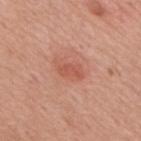follow-up: catalogued during a skin exam; not biopsied | size: ≈3 mm | automated lesion analysis: an area of roughly 3.5 mm², an outline eccentricity of about 0.85 (0 = round, 1 = elongated), and a shape-asymmetry score of about 0.35 (0 = symmetric); an average lesion color of about L≈55 a*≈29 b*≈31 (CIELAB), about 8 CIELAB-L* units darker than the surrounding skin, and a lesion-to-skin contrast of about 5.5 (normalized; higher = more distinct); a color-variation rating of about 1/10; a nevus-likeness score of about 90/100 and a detector confidence of about 100 out of 100 that the crop contains a lesion | patient: male, in their mid- to late 70s | site: the back | image source: total-body-photography crop, ~15 mm field of view | lighting: white-light illumination.